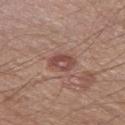The lesion was photographed on a routine skin check and not biopsied; there is no pathology result. The lesion's longest dimension is about 3 mm. A 15 mm crop from a total-body photograph taken for skin-cancer surveillance. An algorithmic analysis of the crop reported an area of roughly 5 mm² and a shape-asymmetry score of about 0.2 (0 = symmetric). The software also gave an average lesion color of about L≈47 a*≈22 b*≈24 (CIELAB), about 10 CIELAB-L* units darker than the surrounding skin, and a normalized lesion–skin contrast near 7.5. The software also gave an automated nevus-likeness rating near 0 out of 100 and lesion-presence confidence of about 100/100. Imaged with white-light lighting. Located on the left thigh. A male patient, aged 63–67.